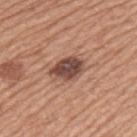Notes:
– biopsy status: no biopsy performed (imaged during a skin exam)
– patient: male, aged 73–77
– anatomic site: the left upper arm
– acquisition: total-body-photography crop, ~15 mm field of view
– illumination: white-light
– diameter: about 4.5 mm
– image-analysis metrics: a lesion area of about 8.5 mm², a shape eccentricity near 0.75, and a symmetry-axis asymmetry near 0.2; lesion-presence confidence of about 100/100